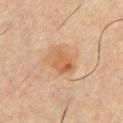Part of a total-body skin-imaging series; this lesion was reviewed on a skin check and was not flagged for biopsy. Captured under cross-polarized illumination. The recorded lesion diameter is about 4 mm. The lesion-visualizer software estimated a footprint of about 8 mm² and two-axis asymmetry of about 0.2. The analysis additionally found a peripheral color-asymmetry measure near 1.5. And it measured an automated nevus-likeness rating near 30 out of 100. The lesion is on the chest. A male patient aged around 60. Cropped from a whole-body photographic skin survey; the tile spans about 15 mm.